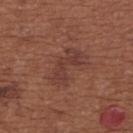biopsy status = imaged on a skin check; not biopsied | anatomic site = the upper back | diameter = ≈4.5 mm | acquisition = ~15 mm crop, total-body skin-cancer survey | subject = female, roughly 65 years of age | tile lighting = white-light illumination.